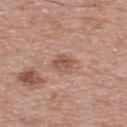Impression: Part of a total-body skin-imaging series; this lesion was reviewed on a skin check and was not flagged for biopsy. Background: A male patient aged 63–67. Captured under white-light illumination. A 15 mm close-up extracted from a 3D total-body photography capture. On the upper back. Approximately 2.5 mm at its widest.